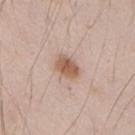Q: Is there a histopathology result?
A: total-body-photography surveillance lesion; no biopsy
Q: What kind of image is this?
A: ~15 mm crop, total-body skin-cancer survey
Q: What are the patient's age and sex?
A: male, in their mid-40s
Q: Lesion location?
A: the right upper arm
Q: What lighting was used for the tile?
A: white-light
Q: Automated lesion metrics?
A: an average lesion color of about L≈58 a*≈19 b*≈29 (CIELAB) and a lesion-to-skin contrast of about 8.5 (normalized; higher = more distinct)